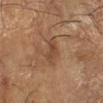| feature | finding |
|---|---|
| workup | imaged on a skin check; not biopsied |
| subject | male, aged approximately 65 |
| TBP lesion metrics | a lesion area of about 4 mm², an outline eccentricity of about 0.85 (0 = round, 1 = elongated), and two-axis asymmetry of about 0.4; a lesion color around L≈45 a*≈20 b*≈31 in CIELAB and a lesion–skin lightness drop of about 8; an automated nevus-likeness rating near 10 out of 100 and lesion-presence confidence of about 100/100 |
| imaging modality | 15 mm crop, total-body photography |
| location | the right forearm |
| size | ~3 mm (longest diameter) |